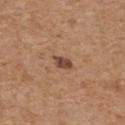This lesion was catalogued during total-body skin photography and was not selected for biopsy. A male subject approximately 70 years of age. This is a white-light tile. The lesion is located on the back. Cropped from a total-body skin-imaging series; the visible field is about 15 mm.